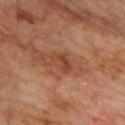Clinical impression:
The lesion was photographed on a routine skin check and not biopsied; there is no pathology result.
Context:
A male patient approximately 70 years of age. A lesion tile, about 15 mm wide, cut from a 3D total-body photograph. From the upper back.